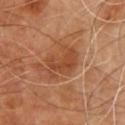Context:
The lesion is on the front of the torso. Measured at roughly 5 mm in maximum diameter. Captured under cross-polarized illumination. A region of skin cropped from a whole-body photographic capture, roughly 15 mm wide. The patient is a male aged around 55.